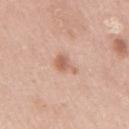biopsy_status: not biopsied; imaged during a skin examination
image:
  source: total-body photography crop
  field_of_view_mm: 15
site: right upper arm
patient:
  sex: male
  age_approx: 50